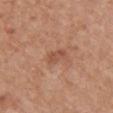follow-up = catalogued during a skin exam; not biopsied
acquisition = ~15 mm tile from a whole-body skin photo
patient = female, aged 68–72
site = the front of the torso
illumination = white-light
diameter = ~3 mm (longest diameter)